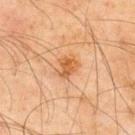follow-up = no biopsy performed (imaged during a skin exam); site = the upper back; imaging modality = total-body-photography crop, ~15 mm field of view; subject = male, in their mid-40s.